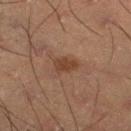{
  "biopsy_status": "not biopsied; imaged during a skin examination",
  "patient": {
    "sex": "male",
    "age_approx": 55
  },
  "lighting": "cross-polarized",
  "image": {
    "source": "total-body photography crop",
    "field_of_view_mm": 15
  },
  "lesion_size": {
    "long_diameter_mm_approx": 3.0
  },
  "site": "leg"
}